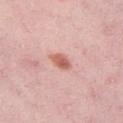Clinical impression: This lesion was catalogued during total-body skin photography and was not selected for biopsy. Context: The lesion is located on the leg. A lesion tile, about 15 mm wide, cut from a 3D total-body photograph. This is a white-light tile. A female patient, about 45 years old. The total-body-photography lesion software estimated a mean CIELAB color near L≈61 a*≈27 b*≈28 and roughly 13 lightness units darker than nearby skin.